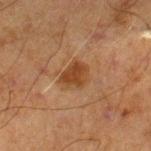workup=no biopsy performed (imaged during a skin exam) | lighting=cross-polarized | diameter=about 3 mm | location=the right lower leg | patient=male, in their 70s | image source=~15 mm tile from a whole-body skin photo.